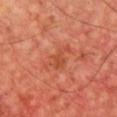acquisition=15 mm crop, total-body photography; patient=male, in their mid-60s; location=the chest; illumination=cross-polarized illumination; lesion size=about 4.5 mm.